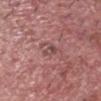Captured during whole-body skin photography for melanoma surveillance; the lesion was not biopsied. A male subject aged 73–77. The lesion is located on the head or neck. A 15 mm close-up tile from a total-body photography series done for melanoma screening.